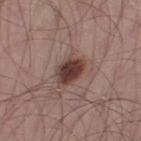Findings:
- follow-up: total-body-photography surveillance lesion; no biopsy
- lighting: white-light illumination
- body site: the right thigh
- lesion size: ≈4 mm
- imaging modality: total-body-photography crop, ~15 mm field of view
- subject: male, roughly 55 years of age